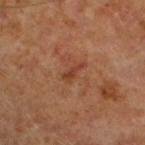{"biopsy_status": "not biopsied; imaged during a skin examination", "automated_metrics": {"cielab_L": 38, "cielab_a": 25, "cielab_b": 30, "vs_skin_darker_L": 7.0, "vs_skin_contrast_norm": 6.5}, "site": "leg", "patient": {"sex": "male", "age_approx": 60}, "lesion_size": {"long_diameter_mm_approx": 2.5}, "image": {"source": "total-body photography crop", "field_of_view_mm": 15}}